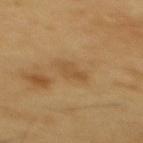Part of a total-body skin-imaging series; this lesion was reviewed on a skin check and was not flagged for biopsy.
The recorded lesion diameter is about 3 mm.
On the back.
A 15 mm close-up tile from a total-body photography series done for melanoma screening.
Automated tile analysis of the lesion measured a lesion area of about 4.5 mm² and a shape-asymmetry score of about 0.25 (0 = symmetric). The software also gave border irregularity of about 2.5 on a 0–10 scale. And it measured lesion-presence confidence of about 100/100.
The patient is a male approximately 60 years of age.
The tile uses cross-polarized illumination.